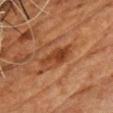biopsy status = imaged on a skin check; not biopsied
image-analysis metrics = a border-irregularity index near 3.5/10, internal color variation of about 3 on a 0–10 scale, and a peripheral color-asymmetry measure near 1
image source = ~15 mm tile from a whole-body skin photo
site = the chest
size = ~4 mm (longest diameter)
patient = male, approximately 65 years of age